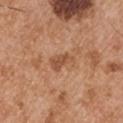Cropped from a total-body skin-imaging series; the visible field is about 15 mm.
A male patient in their mid-50s.
Located on the arm.
The recorded lesion diameter is about 3 mm.